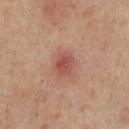{"biopsy_status": "not biopsied; imaged during a skin examination", "lesion_size": {"long_diameter_mm_approx": 2.5}, "site": "mid back", "automated_metrics": {"area_mm2_approx": 5.0, "eccentricity": 0.55, "shape_asymmetry": 0.2, "border_irregularity_0_10": 1.5, "color_variation_0_10": 2.5, "peripheral_color_asymmetry": 1.0, "nevus_likeness_0_100": 10, "lesion_detection_confidence_0_100": 100}, "image": {"source": "total-body photography crop", "field_of_view_mm": 15}, "patient": {"sex": "male", "age_approx": 65}}